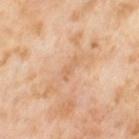The lesion was photographed on a routine skin check and not biopsied; there is no pathology result. A female subject aged approximately 55. A lesion tile, about 15 mm wide, cut from a 3D total-body photograph. The total-body-photography lesion software estimated an eccentricity of roughly 0.95 and a shape-asymmetry score of about 0.4 (0 = symmetric). The lesion is on the left thigh.